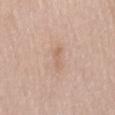Notes:
– follow-up · imaged on a skin check; not biopsied
– body site · the mid back
– imaging modality · 15 mm crop, total-body photography
– image-analysis metrics · an outline eccentricity of about 0.95 (0 = round, 1 = elongated); border irregularity of about 4.5 on a 0–10 scale, a color-variation rating of about 0/10, and radial color variation of about 0
– lesion diameter · about 3 mm
– illumination · white-light
– patient · female, aged approximately 75